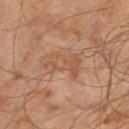The lesion was tiled from a total-body skin photograph and was not biopsied.
A male subject aged 63 to 67.
Cropped from a whole-body photographic skin survey; the tile spans about 15 mm.
The recorded lesion diameter is about 4 mm.
Imaged with cross-polarized lighting.